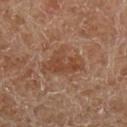Captured during whole-body skin photography for melanoma surveillance; the lesion was not biopsied. The subject is a male aged 53 to 57. Automated tile analysis of the lesion measured a lesion area of about 11 mm², an eccentricity of roughly 0.8, and a symmetry-axis asymmetry near 0.35. The analysis additionally found border irregularity of about 4 on a 0–10 scale, a within-lesion color-variation index near 3.5/10, and radial color variation of about 1. And it measured an automated nevus-likeness rating near 0 out of 100 and lesion-presence confidence of about 100/100. Cropped from a whole-body photographic skin survey; the tile spans about 15 mm. On the left lower leg. The recorded lesion diameter is about 5 mm.